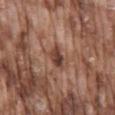Clinical impression:
The lesion was tiled from a total-body skin photograph and was not biopsied.
Image and clinical context:
A 15 mm crop from a total-body photograph taken for skin-cancer surveillance. The lesion is located on the mid back. Approximately 2.5 mm at its widest. This is a white-light tile. A male patient about 75 years old.